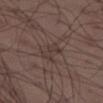Captured during whole-body skin photography for melanoma surveillance; the lesion was not biopsied. The lesion's longest dimension is about 3 mm. A male patient aged 38–42. On the leg. A roughly 15 mm field-of-view crop from a total-body skin photograph.